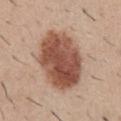Assessment: The lesion was photographed on a routine skin check and not biopsied; there is no pathology result. Acquisition and patient details: A male patient, approximately 30 years of age. From the abdomen. The recorded lesion diameter is about 7.5 mm. Imaged with white-light lighting. A lesion tile, about 15 mm wide, cut from a 3D total-body photograph.